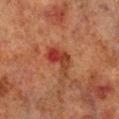workup: imaged on a skin check; not biopsied | location: the leg | acquisition: ~15 mm crop, total-body skin-cancer survey | tile lighting: cross-polarized illumination | image-analysis metrics: an area of roughly 7 mm², an outline eccentricity of about 0.8 (0 = round, 1 = elongated), and a shape-asymmetry score of about 0.4 (0 = symmetric); an average lesion color of about L≈33 a*≈27 b*≈29 (CIELAB), roughly 9 lightness units darker than nearby skin, and a normalized lesion–skin contrast near 8; a border-irregularity rating of about 4.5/10, a color-variation rating of about 7.5/10, and radial color variation of about 2.5 | subject: male, roughly 70 years of age | lesion diameter: ≈4 mm.